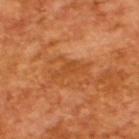Notes:
• biopsy status: total-body-photography surveillance lesion; no biopsy
• tile lighting: cross-polarized illumination
• TBP lesion metrics: an average lesion color of about L≈48 a*≈28 b*≈42 (CIELAB), about 6 CIELAB-L* units darker than the surrounding skin, and a normalized border contrast of about 5; a border-irregularity index near 7.5/10 and radial color variation of about 0.5
• subject: male, aged approximately 65
• lesion size: ≈4.5 mm
• image: total-body-photography crop, ~15 mm field of view